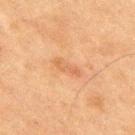On the mid back. This image is a 15 mm lesion crop taken from a total-body photograph. A male subject, aged approximately 75. The total-body-photography lesion software estimated a footprint of about 4 mm² and a shape-asymmetry score of about 0.25 (0 = symmetric). It also reported roughly 6 lightness units darker than nearby skin and a normalized border contrast of about 5. It also reported a classifier nevus-likeness of about 0/100. Imaged with cross-polarized lighting.